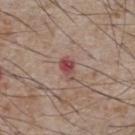Case summary:
* biopsy status: total-body-photography surveillance lesion; no biopsy
* subject: male, aged around 75
* site: the chest
* image source: 15 mm crop, total-body photography